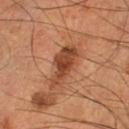biopsy_status: not biopsied; imaged during a skin examination
site: leg
image:
  source: total-body photography crop
  field_of_view_mm: 15
patient:
  sex: male
  age_approx: 55
lesion_size:
  long_diameter_mm_approx: 6.0
lighting: cross-polarized